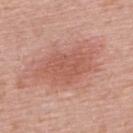biopsy status: imaged on a skin check; not biopsied | image-analysis metrics: a lesion area of about 20 mm² and an outline eccentricity of about 0.85 (0 = round, 1 = elongated) | diameter: ≈7 mm | tile lighting: white-light | patient: female, aged 58–62 | location: the back | acquisition: 15 mm crop, total-body photography.